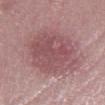{
  "image": {
    "source": "total-body photography crop",
    "field_of_view_mm": 15
  },
  "automated_metrics": {
    "area_mm2_approx": 36.0,
    "eccentricity": 0.8,
    "shape_asymmetry": 0.15,
    "border_irregularity_0_10": 2.5,
    "color_variation_0_10": 3.5,
    "peripheral_color_asymmetry": 1.0,
    "lesion_detection_confidence_0_100": 100
  },
  "lesion_size": {
    "long_diameter_mm_approx": 9.0
  },
  "lighting": "white-light",
  "site": "leg",
  "patient": {
    "sex": "female",
    "age_approx": 45
  }
}